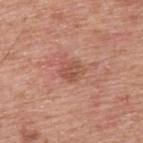Impression: The lesion was tiled from a total-body skin photograph and was not biopsied. Clinical summary: Longest diameter approximately 3 mm. The subject is a male aged around 55. This is a white-light tile. The total-body-photography lesion software estimated an outline eccentricity of about 0.55 (0 = round, 1 = elongated) and a symmetry-axis asymmetry near 0.2. The analysis additionally found an average lesion color of about L≈53 a*≈24 b*≈29 (CIELAB), a lesion–skin lightness drop of about 8, and a normalized lesion–skin contrast near 6. The software also gave a classifier nevus-likeness of about 5/100 and lesion-presence confidence of about 100/100. The lesion is located on the upper back. Cropped from a total-body skin-imaging series; the visible field is about 15 mm.